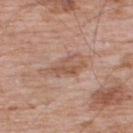  biopsy_status: not biopsied; imaged during a skin examination
  site: upper back
  lighting: white-light
  patient:
    sex: male
    age_approx: 60
  image:
    source: total-body photography crop
    field_of_view_mm: 15
  lesion_size:
    long_diameter_mm_approx: 5.0